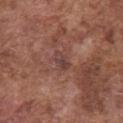<tbp_lesion>
<biopsy_status>not biopsied; imaged during a skin examination</biopsy_status>
<lesion_size>
  <long_diameter_mm_approx>2.5</long_diameter_mm_approx>
</lesion_size>
<site>chest</site>
<lighting>white-light</lighting>
<patient>
  <sex>male</sex>
  <age_approx>75</age_approx>
</patient>
<image>
  <source>total-body photography crop</source>
  <field_of_view_mm>15</field_of_view_mm>
</image>
</tbp_lesion>